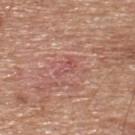Clinical impression:
The lesion was tiled from a total-body skin photograph and was not biopsied.
Acquisition and patient details:
Cropped from a whole-body photographic skin survey; the tile spans about 15 mm. From the upper back. A male patient aged around 65.